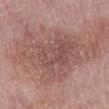* follow-up — no biopsy performed (imaged during a skin exam)
* image source — ~15 mm tile from a whole-body skin photo
* lesion size — ~11.5 mm (longest diameter)
* location — the abdomen
* image-analysis metrics — a lesion area of about 48 mm² and two-axis asymmetry of about 0.45; a mean CIELAB color near L≈52 a*≈20 b*≈23 and about 9 CIELAB-L* units darker than the surrounding skin; a border-irregularity rating of about 7.5/10, a within-lesion color-variation index near 4/10, and radial color variation of about 1.5
* illumination — white-light
* subject — male, aged 73–77